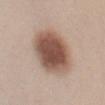  biopsy_status: not biopsied; imaged during a skin examination
  image:
    source: total-body photography crop
    field_of_view_mm: 15
  patient:
    sex: female
    age_approx: 25
  site: chest
  automated_metrics:
    nevus_likeness_0_100: 100
    lesion_detection_confidence_0_100: 100
  lighting: white-light